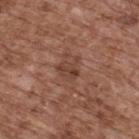Impression:
No biopsy was performed on this lesion — it was imaged during a full skin examination and was not determined to be concerning.
Image and clinical context:
A male patient, approximately 75 years of age. A 15 mm close-up extracted from a 3D total-body photography capture. Located on the upper back. About 3 mm across. This is a white-light tile.